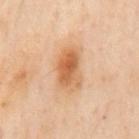Impression:
Imaged during a routine full-body skin examination; the lesion was not biopsied and no histopathology is available.
Image and clinical context:
Imaged with cross-polarized lighting. The subject is a male roughly 55 years of age. A 15 mm close-up tile from a total-body photography series done for melanoma screening. The lesion-visualizer software estimated a lesion area of about 13 mm², a shape eccentricity near 0.8, and two-axis asymmetry of about 0.25. It also reported border irregularity of about 2.5 on a 0–10 scale and internal color variation of about 7 on a 0–10 scale. The software also gave a nevus-likeness score of about 85/100 and lesion-presence confidence of about 100/100. The lesion is on the mid back. Measured at roughly 5 mm in maximum diameter.